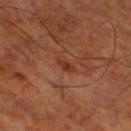Impression:
The lesion was tiled from a total-body skin photograph and was not biopsied.
Acquisition and patient details:
A close-up tile cropped from a whole-body skin photograph, about 15 mm across. Captured under cross-polarized illumination. The patient is a male aged 68–72. Located on the right thigh.